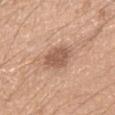biopsy status — imaged on a skin check; not biopsied | patient — male, aged around 20 | acquisition — total-body-photography crop, ~15 mm field of view | image-analysis metrics — an average lesion color of about L≈56 a*≈20 b*≈29 (CIELAB), a lesion–skin lightness drop of about 11, and a normalized lesion–skin contrast near 7.5 | anatomic site — the right upper arm | tile lighting — white-light | lesion diameter — about 3.5 mm.